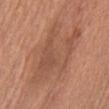{"biopsy_status": "not biopsied; imaged during a skin examination"}